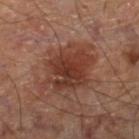Notes:
- biopsy status: total-body-photography surveillance lesion; no biopsy
- diameter: ~6.5 mm (longest diameter)
- anatomic site: the right thigh
- imaging modality: total-body-photography crop, ~15 mm field of view
- TBP lesion metrics: a footprint of about 23 mm² and two-axis asymmetry of about 0.25
- tile lighting: cross-polarized